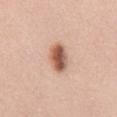Recorded during total-body skin imaging; not selected for excision or biopsy.
On the mid back.
A 15 mm close-up extracted from a 3D total-body photography capture.
A female subject, roughly 40 years of age.